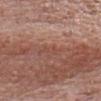Q: Was a biopsy performed?
A: total-body-photography surveillance lesion; no biopsy
Q: Lesion size?
A: about 2.5 mm
Q: What did automated image analysis measure?
A: a lesion area of about 2 mm² and an outline eccentricity of about 0.95 (0 = round, 1 = elongated); a border-irregularity index near 3.5/10, a color-variation rating of about 0/10, and a peripheral color-asymmetry measure near 0; a detector confidence of about 70 out of 100 that the crop contains a lesion
Q: Illumination type?
A: white-light
Q: What kind of image is this?
A: ~15 mm crop, total-body skin-cancer survey
Q: Who is the patient?
A: male, in their mid-60s
Q: Where on the body is the lesion?
A: the head or neck